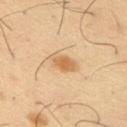Clinical summary:
Located on the left upper arm. A male subject, approximately 40 years of age. Imaged with cross-polarized lighting. The lesion's longest dimension is about 3.5 mm. A lesion tile, about 15 mm wide, cut from a 3D total-body photograph.